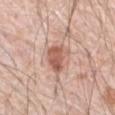notes=total-body-photography surveillance lesion; no biopsy
image=total-body-photography crop, ~15 mm field of view
patient=male, aged around 80
anatomic site=the abdomen